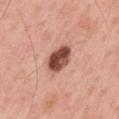The lesion was tiled from a total-body skin photograph and was not biopsied. Automated image analysis of the tile measured a footprint of about 8 mm², a shape eccentricity near 0.7, and a shape-asymmetry score of about 0.2 (0 = symmetric). The analysis additionally found a mean CIELAB color near L≈49 a*≈26 b*≈27 and a lesion–skin lightness drop of about 20. It also reported a border-irregularity index near 1.5/10, internal color variation of about 6.5 on a 0–10 scale, and radial color variation of about 2.5. The lesion's longest dimension is about 3.5 mm. A male patient, aged around 60. The lesion is on the mid back. Captured under white-light illumination. A 15 mm crop from a total-body photograph taken for skin-cancer surveillance.